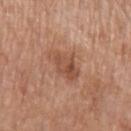This lesion was catalogued during total-body skin photography and was not selected for biopsy. A male patient, roughly 65 years of age. On the left upper arm. Captured under white-light illumination. A 15 mm close-up tile from a total-body photography series done for melanoma screening. Approximately 3.5 mm at its widest.